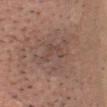This is a white-light tile. The lesion is on the head or neck. Cropped from a whole-body photographic skin survey; the tile spans about 15 mm. Measured at roughly 6.5 mm in maximum diameter. The subject is a male aged approximately 60.